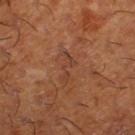Case summary:
- workup — total-body-photography surveillance lesion; no biopsy
- site — the right lower leg
- lighting — cross-polarized
- automated metrics — a mean CIELAB color near L≈41 a*≈23 b*≈30, a lesion–skin lightness drop of about 6, and a normalized border contrast of about 5.5; an automated nevus-likeness rating near 0 out of 100
- lesion size — ≈3.5 mm
- patient — male, aged approximately 65
- image — ~15 mm tile from a whole-body skin photo